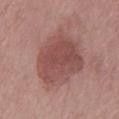{"biopsy_status": "not biopsied; imaged during a skin examination", "image": {"source": "total-body photography crop", "field_of_view_mm": 15}, "site": "chest", "patient": {"sex": "male", "age_approx": 65}}